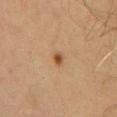{"patient": {"sex": "male", "age_approx": 65}, "site": "front of the torso", "image": {"source": "total-body photography crop", "field_of_view_mm": 15}}